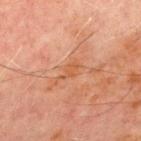Assessment: The lesion was tiled from a total-body skin photograph and was not biopsied. Image and clinical context: A 15 mm close-up extracted from a 3D total-body photography capture. From the chest. The patient is a male aged 68–72.